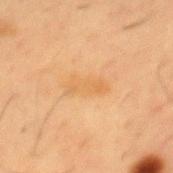The lesion was photographed on a routine skin check and not biopsied; there is no pathology result. About 4.5 mm across. Located on the chest. Imaged with cross-polarized lighting. The patient is a male approximately 55 years of age. Cropped from a whole-body photographic skin survey; the tile spans about 15 mm.